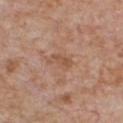biopsy status = catalogued during a skin exam; not biopsied
site = the chest
image source = ~15 mm tile from a whole-body skin photo
subject = male, aged approximately 65
size = ~3 mm (longest diameter)
illumination = white-light